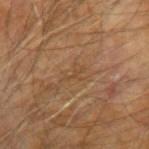imaging modality: ~15 mm tile from a whole-body skin photo | illumination: cross-polarized illumination | site: the left arm | patient: male, aged approximately 60.